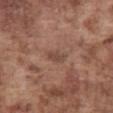Impression: Recorded during total-body skin imaging; not selected for excision or biopsy. Background: A male patient aged approximately 75. The tile uses white-light illumination. Located on the abdomen. Approximately 2.5 mm at its widest. A region of skin cropped from a whole-body photographic capture, roughly 15 mm wide.